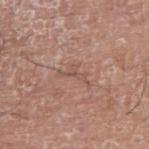Notes:
– follow-up: no biopsy performed (imaged during a skin exam)
– illumination: white-light illumination
– subject: male, in their mid-70s
– diameter: ≈3.5 mm
– imaging modality: ~15 mm tile from a whole-body skin photo
– anatomic site: the right upper arm